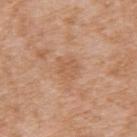  patient:
    sex: female
    age_approx: 40
  site: upper back
  image:
    source: total-body photography crop
    field_of_view_mm: 15